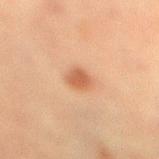No biopsy was performed on this lesion — it was imaged during a full skin examination and was not determined to be concerning.
The lesion-visualizer software estimated a lesion color around L≈47 a*≈21 b*≈31 in CIELAB, a lesion–skin lightness drop of about 10, and a normalized lesion–skin contrast near 7.5.
This image is a 15 mm lesion crop taken from a total-body photograph.
The lesion is located on the right lower leg.
The patient is a female approximately 55 years of age.
The tile uses cross-polarized illumination.
The recorded lesion diameter is about 3 mm.